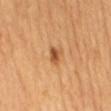workup: total-body-photography surveillance lesion; no biopsy
imaging modality: total-body-photography crop, ~15 mm field of view
site: the mid back
subject: female